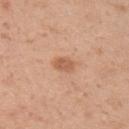Impression: The lesion was photographed on a routine skin check and not biopsied; there is no pathology result. Image and clinical context: A female patient aged 38–42. The tile uses white-light illumination. A 15 mm crop from a total-body photograph taken for skin-cancer surveillance. The total-body-photography lesion software estimated a mean CIELAB color near L≈59 a*≈23 b*≈34, about 10 CIELAB-L* units darker than the surrounding skin, and a normalized border contrast of about 6.5. The analysis additionally found a border-irregularity index near 2/10, a color-variation rating of about 1.5/10, and radial color variation of about 0.5. It also reported an automated nevus-likeness rating near 40 out of 100. About 2.5 mm across. The lesion is located on the right upper arm.